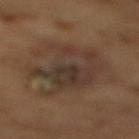The lesion was photographed on a routine skin check and not biopsied; there is no pathology result.
On the mid back.
The patient is a male about 85 years old or older.
A lesion tile, about 15 mm wide, cut from a 3D total-body photograph.
The lesion's longest dimension is about 7.5 mm.
Imaged with cross-polarized lighting.